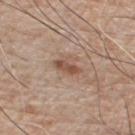| field | value |
|---|---|
| workup | catalogued during a skin exam; not biopsied |
| diameter | ≈3 mm |
| subject | male, roughly 75 years of age |
| image | 15 mm crop, total-body photography |
| body site | the chest |